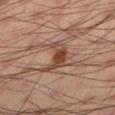<tbp_lesion>
  <biopsy_status>not biopsied; imaged during a skin examination</biopsy_status>
  <automated_metrics>
    <area_mm2_approx>7.5</area_mm2_approx>
    <eccentricity>0.7</eccentricity>
    <shape_asymmetry>0.6</shape_asymmetry>
    <nevus_likeness_0_100>75</nevus_likeness_0_100>
    <lesion_detection_confidence_0_100>100</lesion_detection_confidence_0_100>
  </automated_metrics>
  <lesion_size>
    <long_diameter_mm_approx>3.5</long_diameter_mm_approx>
  </lesion_size>
  <image>
    <source>total-body photography crop</source>
    <field_of_view_mm>15</field_of_view_mm>
  </image>
  <patient>
    <sex>male</sex>
    <age_approx>35</age_approx>
  </patient>
  <site>left lower leg</site>
  <lighting>cross-polarized</lighting>
</tbp_lesion>